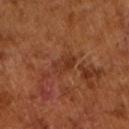notes = no biopsy performed (imaged during a skin exam); image = 15 mm crop, total-body photography; tile lighting = cross-polarized illumination; subject = male, roughly 65 years of age.